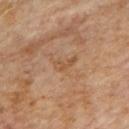biopsy status: no biopsy performed (imaged during a skin exam)
illumination: cross-polarized
patient: male, about 85 years old or older
body site: the upper back
imaging modality: total-body-photography crop, ~15 mm field of view
lesion diameter: ≈3 mm
automated metrics: a lesion area of about 3 mm², an outline eccentricity of about 0.9 (0 = round, 1 = elongated), and a shape-asymmetry score of about 0.5 (0 = symmetric); a lesion color around L≈50 a*≈19 b*≈34 in CIELAB and about 6 CIELAB-L* units darker than the surrounding skin; border irregularity of about 5.5 on a 0–10 scale, a within-lesion color-variation index near 0/10, and a peripheral color-asymmetry measure near 0